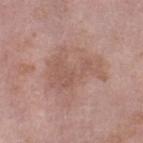The lesion was tiled from a total-body skin photograph and was not biopsied.
Automated image analysis of the tile measured an area of roughly 16 mm², an eccentricity of roughly 0.8, and two-axis asymmetry of about 0.6.
From the right lower leg.
Cropped from a whole-body photographic skin survey; the tile spans about 15 mm.
Captured under white-light illumination.
A female patient, aged around 70.